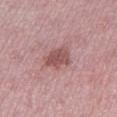<tbp_lesion>
<biopsy_status>not biopsied; imaged during a skin examination</biopsy_status>
<patient>
  <sex>female</sex>
  <age_approx>45</age_approx>
</patient>
<lesion_size>
  <long_diameter_mm_approx>3.5</long_diameter_mm_approx>
</lesion_size>
<site>leg</site>
<image>
  <source>total-body photography crop</source>
  <field_of_view_mm>15</field_of_view_mm>
</image>
<lighting>white-light</lighting>
</tbp_lesion>